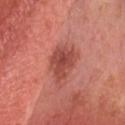Clinical summary:
A male subject aged around 40. Imaged with white-light lighting. On the head or neck. Cropped from a whole-body photographic skin survey; the tile spans about 15 mm. Approximately 4 mm at its widest. An algorithmic analysis of the crop reported a footprint of about 9 mm², an eccentricity of roughly 0.55, and a shape-asymmetry score of about 0.35 (0 = symmetric). The analysis additionally found a lesion-detection confidence of about 100/100.